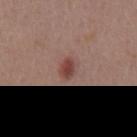  biopsy_status: not biopsied; imaged during a skin examination
  site: mid back
  lighting: white-light
  lesion_size:
    long_diameter_mm_approx: 3.0
  image:
    source: total-body photography crop
    field_of_view_mm: 15
  patient:
    sex: male
    age_approx: 55